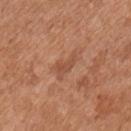{
  "biopsy_status": "not biopsied; imaged during a skin examination",
  "lighting": "white-light",
  "site": "chest",
  "image": {
    "source": "total-body photography crop",
    "field_of_view_mm": 15
  },
  "lesion_size": {
    "long_diameter_mm_approx": 3.5
  },
  "patient": {
    "sex": "male",
    "age_approx": 55
  }
}